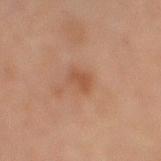<tbp_lesion>
<biopsy_status>not biopsied; imaged during a skin examination</biopsy_status>
<site>right lower leg</site>
<automated_metrics>
  <area_mm2_approx>3.5</area_mm2_approx>
  <eccentricity>0.8</eccentricity>
  <shape_asymmetry>0.25</shape_asymmetry>
  <vs_skin_darker_L>7.0</vs_skin_darker_L>
  <vs_skin_contrast_norm>6.0</vs_skin_contrast_norm>
</automated_metrics>
<image>
  <source>total-body photography crop</source>
  <field_of_view_mm>15</field_of_view_mm>
</image>
<lighting>cross-polarized</lighting>
<lesion_size>
  <long_diameter_mm_approx>2.5</long_diameter_mm_approx>
</lesion_size>
<patient>
  <sex>female</sex>
  <age_approx>70</age_approx>
</patient>
</tbp_lesion>